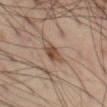notes=total-body-photography surveillance lesion; no biopsy
image=~15 mm tile from a whole-body skin photo
subject=male, about 55 years old
site=the left thigh